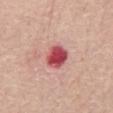This lesion was catalogued during total-body skin photography and was not selected for biopsy. On the back. Automated image analysis of the tile measured an area of roughly 7 mm², a shape eccentricity near 0.3, and two-axis asymmetry of about 0.2. The analysis additionally found a border-irregularity index near 1.5/10, a within-lesion color-variation index near 4.5/10, and peripheral color asymmetry of about 1. And it measured lesion-presence confidence of about 100/100. Cropped from a total-body skin-imaging series; the visible field is about 15 mm. Longest diameter approximately 3 mm. Imaged with white-light lighting. The subject is a male roughly 70 years of age.